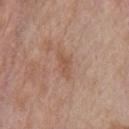A 15 mm close-up extracted from a 3D total-body photography capture. A male patient, aged around 55. Imaged with white-light lighting. On the chest.